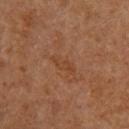Case summary:
• follow-up: catalogued during a skin exam; not biopsied